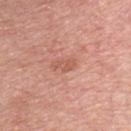Recorded during total-body skin imaging; not selected for excision or biopsy.
Approximately 2.5 mm at its widest.
Located on the chest.
This image is a 15 mm lesion crop taken from a total-body photograph.
A male subject, approximately 45 years of age.